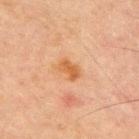notes — no biopsy performed (imaged during a skin exam); subject — male, about 70 years old; site — the chest; lesion diameter — about 3 mm; image — total-body-photography crop, ~15 mm field of view.